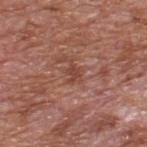| key | value |
|---|---|
| follow-up | catalogued during a skin exam; not biopsied |
| patient | male, about 65 years old |
| imaging modality | ~15 mm crop, total-body skin-cancer survey |
| site | the upper back |
| illumination | white-light illumination |
| TBP lesion metrics | an area of roughly 3 mm², an outline eccentricity of about 0.75 (0 = round, 1 = elongated), and two-axis asymmetry of about 0.5; roughly 8 lightness units darker than nearby skin and a lesion-to-skin contrast of about 6 (normalized; higher = more distinct); a border-irregularity rating of about 5.5/10, a color-variation rating of about 0/10, and peripheral color asymmetry of about 0 |